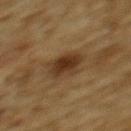{
  "lighting": "cross-polarized",
  "image": {
    "source": "total-body photography crop",
    "field_of_view_mm": 15
  },
  "site": "upper back",
  "lesion_size": {
    "long_diameter_mm_approx": 4.0
  },
  "patient": {
    "sex": "male",
    "age_approx": "85+"
  }
}